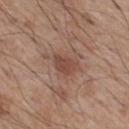Recorded during total-body skin imaging; not selected for excision or biopsy. About 3 mm across. A male subject aged 53–57. Located on the right thigh. Captured under white-light illumination. Automated tile analysis of the lesion measured a border-irregularity rating of about 3/10, internal color variation of about 1.5 on a 0–10 scale, and radial color variation of about 0.5. The analysis additionally found a nevus-likeness score of about 50/100 and lesion-presence confidence of about 100/100. A 15 mm crop from a total-body photograph taken for skin-cancer surveillance.